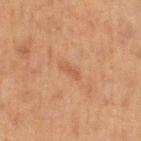notes: imaged on a skin check; not biopsied
diameter: ≈2.5 mm
subject: female, about 40 years old
lighting: cross-polarized
location: the leg
acquisition: ~15 mm crop, total-body skin-cancer survey